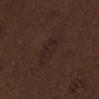Captured during whole-body skin photography for melanoma surveillance; the lesion was not biopsied.
A male subject, about 70 years old.
This is a white-light tile.
The lesion is on the chest.
A region of skin cropped from a whole-body photographic capture, roughly 15 mm wide.
About 5.5 mm across.